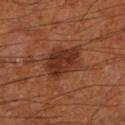{
  "biopsy_status": "not biopsied; imaged during a skin examination",
  "image": {
    "source": "total-body photography crop",
    "field_of_view_mm": 15
  },
  "site": "left lower leg",
  "lighting": "cross-polarized",
  "patient": {
    "sex": "male",
    "age_approx": 65
  },
  "lesion_size": {
    "long_diameter_mm_approx": 5.0
  }
}